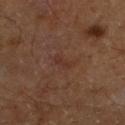biopsy status: imaged on a skin check; not biopsied | body site: the left lower leg | image: total-body-photography crop, ~15 mm field of view | automated lesion analysis: a footprint of about 2.5 mm², a shape eccentricity near 0.95, and a shape-asymmetry score of about 0.35 (0 = symmetric) | patient: male, aged 58 to 62 | tile lighting: cross-polarized illumination | lesion size: about 2.5 mm.